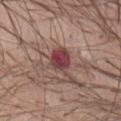Findings:
* size · about 3.5 mm
* patient · female, aged around 50
* anatomic site · the abdomen
* imaging modality · total-body-photography crop, ~15 mm field of view